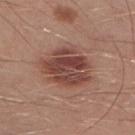Recorded during total-body skin imaging; not selected for excision or biopsy. From the right lower leg. Automated tile analysis of the lesion measured a border-irregularity rating of about 3/10, a within-lesion color-variation index near 5.5/10, and radial color variation of about 2. Approximately 6 mm at its widest. Cropped from a total-body skin-imaging series; the visible field is about 15 mm. The patient is a male about 30 years old.